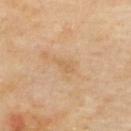{"biopsy_status": "not biopsied; imaged during a skin examination", "patient": {"sex": "male", "age_approx": 65}, "lighting": "cross-polarized", "image": {"source": "total-body photography crop", "field_of_view_mm": 15}, "lesion_size": {"long_diameter_mm_approx": 2.5}, "site": "upper back", "automated_metrics": {"area_mm2_approx": 2.5, "eccentricity": 0.85, "cielab_L": 63, "cielab_a": 17, "cielab_b": 38, "vs_skin_contrast_norm": 5.0, "nevus_likeness_0_100": 0, "lesion_detection_confidence_0_100": 100}}